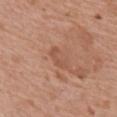Context: Captured under white-light illumination. From the back. A 15 mm close-up tile from a total-body photography series done for melanoma screening. The lesion's longest dimension is about 3 mm. The subject is a female in their mid-70s.